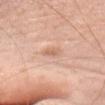{"biopsy_status": "not biopsied; imaged during a skin examination"}